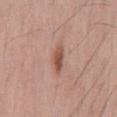{
  "biopsy_status": "not biopsied; imaged during a skin examination",
  "image": {
    "source": "total-body photography crop",
    "field_of_view_mm": 15
  },
  "lesion_size": {
    "long_diameter_mm_approx": 3.5
  },
  "automated_metrics": {
    "area_mm2_approx": 4.0,
    "cielab_L": 52,
    "cielab_a": 23,
    "cielab_b": 28,
    "vs_skin_contrast_norm": 8.5,
    "nevus_likeness_0_100": 100,
    "lesion_detection_confidence_0_100": 100
  },
  "site": "mid back",
  "patient": {
    "sex": "male",
    "age_approx": 55
  },
  "lighting": "white-light"
}